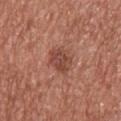This lesion was catalogued during total-body skin photography and was not selected for biopsy. The tile uses white-light illumination. Automated image analysis of the tile measured an area of roughly 7.5 mm², an eccentricity of roughly 0.75, and two-axis asymmetry of about 0.2. The software also gave border irregularity of about 2.5 on a 0–10 scale, internal color variation of about 3.5 on a 0–10 scale, and a peripheral color-asymmetry measure near 1. A male subject approximately 45 years of age. From the chest. Approximately 4 mm at its widest. A roughly 15 mm field-of-view crop from a total-body skin photograph.